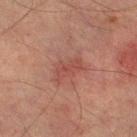Captured during whole-body skin photography for melanoma surveillance; the lesion was not biopsied. On the leg. Measured at roughly 3.5 mm in maximum diameter. This is a cross-polarized tile. A lesion tile, about 15 mm wide, cut from a 3D total-body photograph. The subject is a male in their mid- to late 70s.